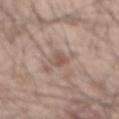Part of a total-body skin-imaging series; this lesion was reviewed on a skin check and was not flagged for biopsy. A 15 mm crop from a total-body photograph taken for skin-cancer surveillance. The subject is a male in their mid-40s. About 2.5 mm across. On the mid back.